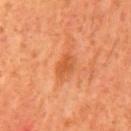{"biopsy_status": "not biopsied; imaged during a skin examination", "site": "mid back", "image": {"source": "total-body photography crop", "field_of_view_mm": 15}, "automated_metrics": {"shape_asymmetry": 0.25, "vs_skin_darker_L": 9.0, "vs_skin_contrast_norm": 6.5, "border_irregularity_0_10": 2.5, "color_variation_0_10": 2.0, "peripheral_color_asymmetry": 0.5, "nevus_likeness_0_100": 20, "lesion_detection_confidence_0_100": 100}, "lesion_size": {"long_diameter_mm_approx": 3.5}, "patient": {"sex": "male", "age_approx": 60}}